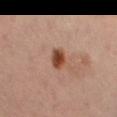{
  "biopsy_status": "not biopsied; imaged during a skin examination",
  "patient": {
    "sex": "female",
    "age_approx": 45
  },
  "lesion_size": {
    "long_diameter_mm_approx": 2.5
  },
  "site": "left thigh",
  "image": {
    "source": "total-body photography crop",
    "field_of_view_mm": 15
  },
  "automated_metrics": {
    "area_mm2_approx": 4.5,
    "shape_asymmetry": 0.15,
    "border_irregularity_0_10": 1.0,
    "color_variation_0_10": 6.0,
    "peripheral_color_asymmetry": 2.0,
    "nevus_likeness_0_100": 100,
    "lesion_detection_confidence_0_100": 100
  },
  "lighting": "cross-polarized"
}